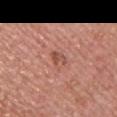{
  "biopsy_status": "not biopsied; imaged during a skin examination",
  "automated_metrics": {
    "area_mm2_approx": 3.0,
    "eccentricity": 0.85,
    "shape_asymmetry": 0.35,
    "cielab_L": 51,
    "cielab_a": 25,
    "cielab_b": 29,
    "vs_skin_darker_L": 9.0,
    "vs_skin_contrast_norm": 6.5,
    "color_variation_0_10": 0.5,
    "peripheral_color_asymmetry": 0.0,
    "nevus_likeness_0_100": 0,
    "lesion_detection_confidence_0_100": 100
  },
  "patient": {
    "sex": "female",
    "age_approx": 45
  },
  "site": "left upper arm",
  "image": {
    "source": "total-body photography crop",
    "field_of_view_mm": 15
  },
  "lighting": "white-light"
}